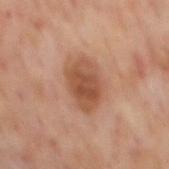<record>
<biopsy_status>not biopsied; imaged during a skin examination</biopsy_status>
</record>